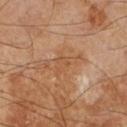This lesion was catalogued during total-body skin photography and was not selected for biopsy. A 15 mm close-up extracted from a 3D total-body photography capture. Measured at roughly 3 mm in maximum diameter. Located on the right lower leg. A male subject aged 58–62.